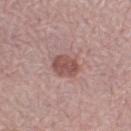Case summary:
- follow-up: catalogued during a skin exam; not biopsied